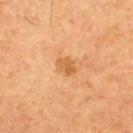| field | value |
|---|---|
| follow-up | total-body-photography surveillance lesion; no biopsy |
| subject | male, in their 60s |
| lesion size | ≈2.5 mm |
| image | ~15 mm crop, total-body skin-cancer survey |
| lighting | cross-polarized illumination |